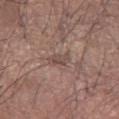Captured during whole-body skin photography for melanoma surveillance; the lesion was not biopsied. A lesion tile, about 15 mm wide, cut from a 3D total-body photograph. A male subject aged 63 to 67. Located on the left forearm. Longest diameter approximately 2.5 mm. The lesion-visualizer software estimated an average lesion color of about L≈47 a*≈16 b*≈22 (CIELAB) and a lesion–skin lightness drop of about 8. And it measured border irregularity of about 2.5 on a 0–10 scale, a color-variation rating of about 2/10, and peripheral color asymmetry of about 0.5. The analysis additionally found a classifier nevus-likeness of about 0/100 and a lesion-detection confidence of about 90/100.